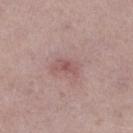Assessment: Recorded during total-body skin imaging; not selected for excision or biopsy. Context: A roughly 15 mm field-of-view crop from a total-body skin photograph. The lesion is located on the right lower leg. A female patient aged 58 to 62. Approximately 3 mm at its widest. An algorithmic analysis of the crop reported a lesion color around L≈53 a*≈22 b*≈21 in CIELAB, about 8 CIELAB-L* units darker than the surrounding skin, and a normalized border contrast of about 6. And it measured a classifier nevus-likeness of about 5/100 and a detector confidence of about 100 out of 100 that the crop contains a lesion.